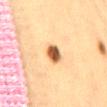Impression: Imaged during a routine full-body skin examination; the lesion was not biopsied and no histopathology is available. Context: The lesion is located on the mid back. An algorithmic analysis of the crop reported an area of roughly 5 mm², an eccentricity of roughly 0.65, and two-axis asymmetry of about 0.2. It also reported a mean CIELAB color near L≈51 a*≈20 b*≈35, roughly 19 lightness units darker than nearby skin, and a normalized lesion–skin contrast near 12.5. This is a cross-polarized tile. A female subject, aged 38–42. A 15 mm close-up tile from a total-body photography series done for melanoma screening. About 3 mm across.